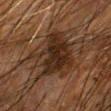No biopsy was performed on this lesion — it was imaged during a full skin examination and was not determined to be concerning. The recorded lesion diameter is about 7 mm. The patient is a male about 65 years old. Cropped from a total-body skin-imaging series; the visible field is about 15 mm. This is a cross-polarized tile. On the left forearm. Automated image analysis of the tile measured an area of roughly 25 mm² and an eccentricity of roughly 0.65. And it measured a border-irregularity rating of about 5.5/10, internal color variation of about 5 on a 0–10 scale, and radial color variation of about 1.5.